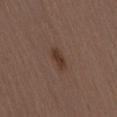Findings:
• follow-up: catalogued during a skin exam; not biopsied
• imaging modality: ~15 mm crop, total-body skin-cancer survey
• anatomic site: the leg
• patient: female, roughly 40 years of age